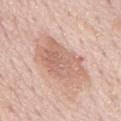Assessment:
The lesion was tiled from a total-body skin photograph and was not biopsied.
Clinical summary:
This is a white-light tile. The patient is a male aged 73 to 77. This image is a 15 mm lesion crop taken from a total-body photograph. The recorded lesion diameter is about 6.5 mm. From the mid back.